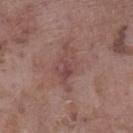{
  "biopsy_status": "not biopsied; imaged during a skin examination",
  "lesion_size": {
    "long_diameter_mm_approx": 5.5
  },
  "lighting": "white-light",
  "site": "left lower leg",
  "image": {
    "source": "total-body photography crop",
    "field_of_view_mm": 15
  },
  "patient": {
    "sex": "male",
    "age_approx": 75
  },
  "automated_metrics": {
    "area_mm2_approx": 10.0,
    "eccentricity": 0.85,
    "shape_asymmetry": 0.35,
    "cielab_L": 46,
    "cielab_a": 20,
    "cielab_b": 21,
    "border_irregularity_0_10": 5.0,
    "color_variation_0_10": 4.5,
    "nevus_likeness_0_100": 0
  }
}